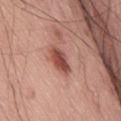No biopsy was performed on this lesion — it was imaged during a full skin examination and was not determined to be concerning.
Imaged with white-light lighting.
On the lower back.
This image is a 15 mm lesion crop taken from a total-body photograph.
Approximately 3.5 mm at its widest.
A male subject, aged 68 to 72.